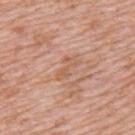notes: total-body-photography surveillance lesion; no biopsy
diameter: ~3.5 mm (longest diameter)
location: the arm
subject: male, aged approximately 50
imaging modality: ~15 mm crop, total-body skin-cancer survey